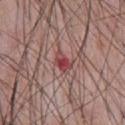Q: Is there a histopathology result?
A: imaged on a skin check; not biopsied
Q: Where on the body is the lesion?
A: the chest
Q: Who is the patient?
A: male, roughly 60 years of age
Q: Automated lesion metrics?
A: a lesion color around L≈44 a*≈29 b*≈21 in CIELAB, roughly 11 lightness units darker than nearby skin, and a normalized border contrast of about 8; a border-irregularity index near 2/10 and internal color variation of about 6.5 on a 0–10 scale; a nevus-likeness score of about 0/100
Q: How was this image acquired?
A: ~15 mm tile from a whole-body skin photo
Q: Lesion size?
A: about 2.5 mm
Q: How was the tile lit?
A: white-light